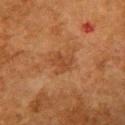No biopsy was performed on this lesion — it was imaged during a full skin examination and was not determined to be concerning. About 2.5 mm across. A female patient about 50 years old. Captured under cross-polarized illumination. The lesion is on the chest. A lesion tile, about 15 mm wide, cut from a 3D total-body photograph.